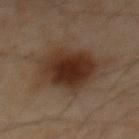Assessment: The lesion was tiled from a total-body skin photograph and was not biopsied. Image and clinical context: About 7 mm across. The lesion is located on the abdomen. A male patient aged 58–62. The tile uses cross-polarized illumination. Cropped from a whole-body photographic skin survey; the tile spans about 15 mm.